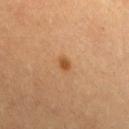Impression:
The lesion was photographed on a routine skin check and not biopsied; there is no pathology result.
Clinical summary:
Longest diameter approximately 2 mm. Captured under cross-polarized illumination. The lesion is located on the front of the torso. A region of skin cropped from a whole-body photographic capture, roughly 15 mm wide. Automated tile analysis of the lesion measured an average lesion color of about L≈48 a*≈21 b*≈37 (CIELAB) and about 9 CIELAB-L* units darker than the surrounding skin. It also reported a border-irregularity rating of about 2.5/10, a within-lesion color-variation index near 0.5/10, and peripheral color asymmetry of about 0. It also reported a classifier nevus-likeness of about 90/100 and lesion-presence confidence of about 100/100. A female subject, aged around 30.